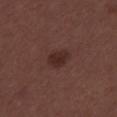The lesion was tiled from a total-body skin photograph and was not biopsied.